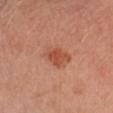<lesion>
<biopsy_status>not biopsied; imaged during a skin examination</biopsy_status>
<site>head or neck</site>
<image>
  <source>total-body photography crop</source>
  <field_of_view_mm>15</field_of_view_mm>
</image>
<automated_metrics>
  <cielab_L>50</cielab_L>
  <cielab_a>26</cielab_a>
  <cielab_b>32</cielab_b>
  <vs_skin_darker_L>8.0</vs_skin_darker_L>
  <peripheral_color_asymmetry>1.0</peripheral_color_asymmetry>
  <lesion_detection_confidence_0_100>100</lesion_detection_confidence_0_100>
</automated_metrics>
<patient>
  <sex>female</sex>
  <age_approx>35</age_approx>
</patient>
</lesion>